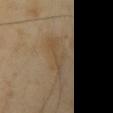{
  "biopsy_status": "not biopsied; imaged during a skin examination"
}